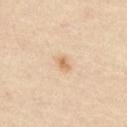This lesion was catalogued during total-body skin photography and was not selected for biopsy. Located on the abdomen. This image is a 15 mm lesion crop taken from a total-body photograph. The total-body-photography lesion software estimated a footprint of about 2 mm² and a symmetry-axis asymmetry near 0.35. The software also gave a lesion color around L≈68 a*≈19 b*≈38 in CIELAB and about 10 CIELAB-L* units darker than the surrounding skin. The software also gave a border-irregularity index near 2.5/10 and internal color variation of about 2 on a 0–10 scale. The analysis additionally found a classifier nevus-likeness of about 80/100. A male patient aged 53 to 57.